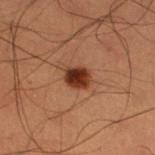lighting: cross-polarized illumination; patient: male, approximately 50 years of age; imaging modality: total-body-photography crop, ~15 mm field of view; anatomic site: the right thigh; lesion diameter: ~3 mm (longest diameter).